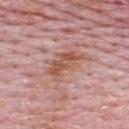biopsy status — imaged on a skin check; not biopsied | patient — male, in their mid- to late 60s | acquisition — ~15 mm tile from a whole-body skin photo | site — the upper back.